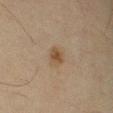– notes — imaged on a skin check; not biopsied
– patient — male, in their mid-60s
– size — about 2.5 mm
– illumination — cross-polarized illumination
– image — ~15 mm crop, total-body skin-cancer survey
– body site — the leg